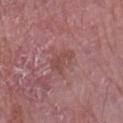{
  "biopsy_status": "not biopsied; imaged during a skin examination",
  "site": "right forearm",
  "image": {
    "source": "total-body photography crop",
    "field_of_view_mm": 15
  },
  "lesion_size": {
    "long_diameter_mm_approx": 3.0
  },
  "automated_metrics": {
    "eccentricity": 0.75,
    "shape_asymmetry": 0.5,
    "cielab_L": 47,
    "cielab_a": 24,
    "cielab_b": 22,
    "vs_skin_darker_L": 7.0,
    "vs_skin_contrast_norm": 5.5,
    "color_variation_0_10": 1.5,
    "nevus_likeness_0_100": 0,
    "lesion_detection_confidence_0_100": 95
  },
  "patient": {
    "sex": "male",
    "age_approx": 75
  },
  "lighting": "white-light"
}